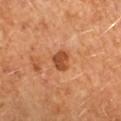follow-up = no biopsy performed (imaged during a skin exam); lesion size = about 2.5 mm; site = the left forearm; image source = total-body-photography crop, ~15 mm field of view; subject = female, in their mid- to late 60s; tile lighting = cross-polarized illumination.